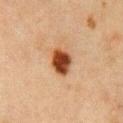The lesion was photographed on a routine skin check and not biopsied; there is no pathology result. The lesion's longest dimension is about 3.5 mm. A female patient about 50 years old. The lesion is located on the chest. The total-body-photography lesion software estimated an area of roughly 7.5 mm², an outline eccentricity of about 0.65 (0 = round, 1 = elongated), and a shape-asymmetry score of about 0.15 (0 = symmetric). And it measured a lesion color around L≈38 a*≈23 b*≈32 in CIELAB, roughly 17 lightness units darker than nearby skin, and a lesion-to-skin contrast of about 13.5 (normalized; higher = more distinct). The analysis additionally found border irregularity of about 1.5 on a 0–10 scale, a within-lesion color-variation index near 5/10, and a peripheral color-asymmetry measure near 1.5. It also reported a classifier nevus-likeness of about 100/100 and a lesion-detection confidence of about 100/100. Captured under cross-polarized illumination. A lesion tile, about 15 mm wide, cut from a 3D total-body photograph.